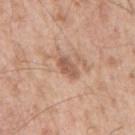Clinical impression:
The lesion was tiled from a total-body skin photograph and was not biopsied.
Acquisition and patient details:
A male subject in their mid- to late 50s. A region of skin cropped from a whole-body photographic capture, roughly 15 mm wide. On the back. Captured under white-light illumination.